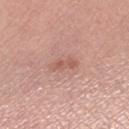The lesion was photographed on a routine skin check and not biopsied; there is no pathology result. On the right thigh. The patient is a female in their mid-50s. A close-up tile cropped from a whole-body skin photograph, about 15 mm across.